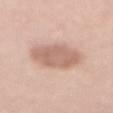Assessment: No biopsy was performed on this lesion — it was imaged during a full skin examination and was not determined to be concerning. Clinical summary: Measured at roughly 6 mm in maximum diameter. A female patient, aged 63–67. On the chest. The lesion-visualizer software estimated a footprint of about 16 mm², an outline eccentricity of about 0.8 (0 = round, 1 = elongated), and two-axis asymmetry of about 0.1. And it measured an average lesion color of about L≈63 a*≈19 b*≈27 (CIELAB) and a normalized lesion–skin contrast near 7. It also reported border irregularity of about 1.5 on a 0–10 scale, a color-variation rating of about 2.5/10, and radial color variation of about 1. Imaged with white-light lighting. Cropped from a total-body skin-imaging series; the visible field is about 15 mm.